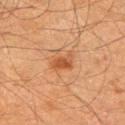The lesion was tiled from a total-body skin photograph and was not biopsied. On the left upper arm. A lesion tile, about 15 mm wide, cut from a 3D total-body photograph. This is a cross-polarized tile. The recorded lesion diameter is about 3 mm. The lesion-visualizer software estimated a lesion area of about 4 mm² and a symmetry-axis asymmetry near 0.4. It also reported a border-irregularity index near 4/10, a color-variation rating of about 2/10, and a peripheral color-asymmetry measure near 0.5. And it measured an automated nevus-likeness rating near 75 out of 100 and a lesion-detection confidence of about 100/100. A male subject about 65 years old.